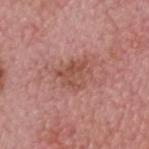Clinical impression:
No biopsy was performed on this lesion — it was imaged during a full skin examination and was not determined to be concerning.
Image and clinical context:
Cropped from a whole-body photographic skin survey; the tile spans about 15 mm. The tile uses white-light illumination. A male subject aged 73–77. On the head or neck. Approximately 3.5 mm at its widest.